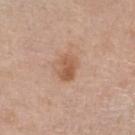No biopsy was performed on this lesion — it was imaged during a full skin examination and was not determined to be concerning. Captured under white-light illumination. From the right forearm. This image is a 15 mm lesion crop taken from a total-body photograph. Longest diameter approximately 3.5 mm. The lesion-visualizer software estimated an area of roughly 5.5 mm², an eccentricity of roughly 0.8, and two-axis asymmetry of about 0.2. The software also gave roughly 10 lightness units darker than nearby skin and a normalized lesion–skin contrast near 7.5. And it measured border irregularity of about 2.5 on a 0–10 scale, a within-lesion color-variation index near 3.5/10, and a peripheral color-asymmetry measure near 1.5. The subject is a female aged approximately 55.